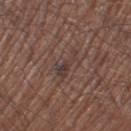Impression:
Imaged during a routine full-body skin examination; the lesion was not biopsied and no histopathology is available.
Acquisition and patient details:
Automated image analysis of the tile measured a footprint of about 5 mm² and an eccentricity of roughly 0.95. The analysis additionally found a mean CIELAB color near L≈37 a*≈16 b*≈19 and a lesion-to-skin contrast of about 6.5 (normalized; higher = more distinct). The software also gave a classifier nevus-likeness of about 0/100 and lesion-presence confidence of about 55/100. Located on the leg. A male patient, in their mid-60s. Measured at roughly 4.5 mm in maximum diameter. This is a white-light tile. A close-up tile cropped from a whole-body skin photograph, about 15 mm across.